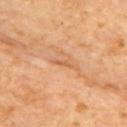Located on the upper back. A female subject approximately 65 years of age. Measured at roughly 3 mm in maximum diameter. Imaged with cross-polarized lighting. Cropped from a total-body skin-imaging series; the visible field is about 15 mm.